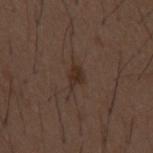Recorded during total-body skin imaging; not selected for excision or biopsy. Imaged with white-light lighting. A roughly 15 mm field-of-view crop from a total-body skin photograph. The patient is a male roughly 50 years of age. About 2.5 mm across. Located on the mid back.